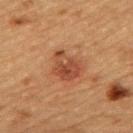Impression:
Part of a total-body skin-imaging series; this lesion was reviewed on a skin check and was not flagged for biopsy.
Background:
The lesion-visualizer software estimated a shape eccentricity near 0.65 and a symmetry-axis asymmetry near 0.3. And it measured a lesion color around L≈39 a*≈23 b*≈30 in CIELAB, about 9 CIELAB-L* units darker than the surrounding skin, and a lesion-to-skin contrast of about 7.5 (normalized; higher = more distinct). The analysis additionally found border irregularity of about 3 on a 0–10 scale, a within-lesion color-variation index near 5/10, and a peripheral color-asymmetry measure near 1.5. The analysis additionally found an automated nevus-likeness rating near 65 out of 100 and a detector confidence of about 100 out of 100 that the crop contains a lesion. The tile uses cross-polarized illumination. A female subject aged approximately 70. A roughly 15 mm field-of-view crop from a total-body skin photograph. On the upper back.